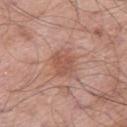| feature | finding |
|---|---|
| location | the right thigh |
| tile lighting | white-light illumination |
| lesion size | ≈3 mm |
| subject | male, aged around 70 |
| image source | ~15 mm tile from a whole-body skin photo |